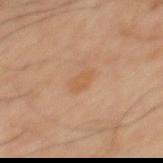Recorded during total-body skin imaging; not selected for excision or biopsy.
On the mid back.
The lesion's longest dimension is about 2.5 mm.
The lesion-visualizer software estimated a normalized lesion–skin contrast near 5.5. It also reported an automated nevus-likeness rating near 10 out of 100 and a detector confidence of about 100 out of 100 that the crop contains a lesion.
A 15 mm close-up tile from a total-body photography series done for melanoma screening.
A male patient, aged 43 to 47.
This is a cross-polarized tile.